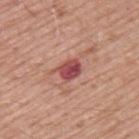workup: total-body-photography surveillance lesion; no biopsy | patient: male, about 70 years old | automated lesion analysis: an average lesion color of about L≈50 a*≈30 b*≈23 (CIELAB) and a normalized lesion–skin contrast near 9.5; a border-irregularity rating of about 3/10; an automated nevus-likeness rating near 5 out of 100 and a detector confidence of about 100 out of 100 that the crop contains a lesion | size: about 3 mm | acquisition: ~15 mm tile from a whole-body skin photo | anatomic site: the upper back | illumination: white-light illumination.